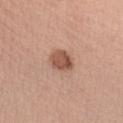<tbp_lesion>
<biopsy_status>not biopsied; imaged during a skin examination</biopsy_status>
<automated_metrics>
  <area_mm2_approx>6.0</area_mm2_approx>
  <eccentricity>0.6</eccentricity>
  <shape_asymmetry>0.15</shape_asymmetry>
  <vs_skin_darker_L>13.0</vs_skin_darker_L>
  <nevus_likeness_0_100>80</nevus_likeness_0_100>
  <lesion_detection_confidence_0_100>100</lesion_detection_confidence_0_100>
</automated_metrics>
<patient>
  <sex>female</sex>
  <age_approx>40</age_approx>
</patient>
<site>left upper arm</site>
<lesion_size>
  <long_diameter_mm_approx>3.0</long_diameter_mm_approx>
</lesion_size>
<lighting>white-light</lighting>
<image>
  <source>total-body photography crop</source>
  <field_of_view_mm>15</field_of_view_mm>
</image>
</tbp_lesion>